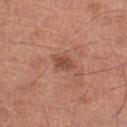– follow-up: catalogued during a skin exam; not biopsied
– subject: male, in their mid- to late 60s
– image source: ~15 mm crop, total-body skin-cancer survey
– location: the left lower leg